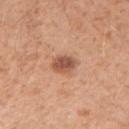No biopsy was performed on this lesion — it was imaged during a full skin examination and was not determined to be concerning.
A lesion tile, about 15 mm wide, cut from a 3D total-body photograph.
Imaged with white-light lighting.
A male subject, aged approximately 30.
The lesion is located on the right upper arm.